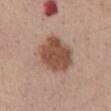Recorded during total-body skin imaging; not selected for excision or biopsy. An algorithmic analysis of the crop reported a mean CIELAB color near L≈50 a*≈21 b*≈28 and a lesion-to-skin contrast of about 10 (normalized; higher = more distinct). A male subject, roughly 55 years of age. The lesion's longest dimension is about 5.5 mm. A lesion tile, about 15 mm wide, cut from a 3D total-body photograph. Located on the front of the torso.